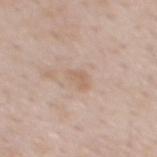The lesion was tiled from a total-body skin photograph and was not biopsied. A male subject, approximately 50 years of age. This is a white-light tile. Cropped from a total-body skin-imaging series; the visible field is about 15 mm. On the mid back. Longest diameter approximately 2.5 mm.